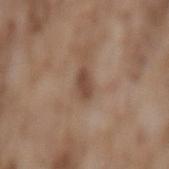notes: catalogued during a skin exam; not biopsied | patient: male, aged around 75 | site: the lower back | image source: ~15 mm tile from a whole-body skin photo | tile lighting: white-light illumination | automated lesion analysis: a footprint of about 3.5 mm² and a shape-asymmetry score of about 0.2 (0 = symmetric); an average lesion color of about L≈45 a*≈18 b*≈27 (CIELAB), roughly 11 lightness units darker than nearby skin, and a normalized lesion–skin contrast near 8; a border-irregularity index near 2/10, a within-lesion color-variation index near 1/10, and peripheral color asymmetry of about 0.5 | diameter: ~3 mm (longest diameter).